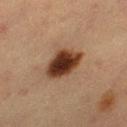This lesion was catalogued during total-body skin photography and was not selected for biopsy.
A female subject aged 53–57.
Cropped from a total-body skin-imaging series; the visible field is about 15 mm.
The lesion's longest dimension is about 4.5 mm.
Located on the left thigh.
An algorithmic analysis of the crop reported an area of roughly 12 mm², a shape eccentricity near 0.65, and a shape-asymmetry score of about 0.15 (0 = symmetric). The software also gave an automated nevus-likeness rating near 100 out of 100 and a lesion-detection confidence of about 100/100.